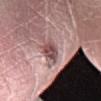- notes: imaged on a skin check; not biopsied
- subject: male, approximately 30 years of age
- lighting: white-light illumination
- location: the left lower leg
- lesion size: ≈3 mm
- acquisition: ~15 mm tile from a whole-body skin photo